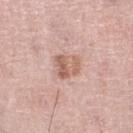Impression: Part of a total-body skin-imaging series; this lesion was reviewed on a skin check and was not flagged for biopsy. Context: A 15 mm close-up extracted from a 3D total-body photography capture. The tile uses white-light illumination. Longest diameter approximately 3 mm. The lesion is on the right lower leg. A male patient aged approximately 65.